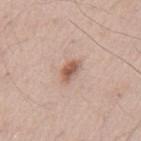Imaged during a routine full-body skin examination; the lesion was not biopsied and no histopathology is available. This is a white-light tile. The patient is a male aged around 55. The lesion is on the mid back. A lesion tile, about 15 mm wide, cut from a 3D total-body photograph.